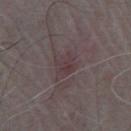<lesion>
<patient>
  <sex>male</sex>
  <age_approx>65</age_approx>
</patient>
<image>
  <source>total-body photography crop</source>
  <field_of_view_mm>15</field_of_view_mm>
</image>
<lighting>white-light</lighting>
<lesion_size>
  <long_diameter_mm_approx>3.5</long_diameter_mm_approx>
</lesion_size>
<automated_metrics>
  <shape_asymmetry>0.25</shape_asymmetry>
  <vs_skin_darker_L>5.0</vs_skin_darker_L>
  <vs_skin_contrast_norm>5.0</vs_skin_contrast_norm>
</automated_metrics>
<site>chest</site>
</lesion>